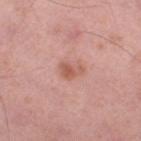Automated image analysis of the tile measured an average lesion color of about L≈58 a*≈25 b*≈28 (CIELAB) and a normalized border contrast of about 6.5. And it measured lesion-presence confidence of about 100/100. A region of skin cropped from a whole-body photographic capture, roughly 15 mm wide. A male patient, aged around 55. The recorded lesion diameter is about 3 mm. The lesion is located on the right thigh.